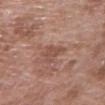No biopsy was performed on this lesion — it was imaged during a full skin examination and was not determined to be concerning. From the arm. A female patient aged around 70. This image is a 15 mm lesion crop taken from a total-body photograph. The lesion-visualizer software estimated an eccentricity of roughly 0.65. The analysis additionally found a border-irregularity index near 4.5/10 and a peripheral color-asymmetry measure near 0.5. The recorded lesion diameter is about 2.5 mm.